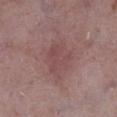Q: Was a biopsy performed?
A: imaged on a skin check; not biopsied
Q: Lesion location?
A: the right lower leg
Q: What kind of image is this?
A: ~15 mm tile from a whole-body skin photo
Q: Illumination type?
A: white-light illumination
Q: What are the patient's age and sex?
A: male, aged around 75